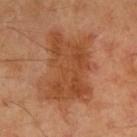Impression: Imaged during a routine full-body skin examination; the lesion was not biopsied and no histopathology is available. Background: A male patient approximately 45 years of age. The total-body-photography lesion software estimated a lesion area of about 35 mm². The software also gave a lesion–skin lightness drop of about 9 and a normalized border contrast of about 7. It also reported a border-irregularity index near 5.5/10, a color-variation rating of about 4/10, and radial color variation of about 1.5. The software also gave a classifier nevus-likeness of about 0/100 and lesion-presence confidence of about 100/100. Captured under cross-polarized illumination. A 15 mm close-up tile from a total-body photography series done for melanoma screening. On the right upper arm. The recorded lesion diameter is about 8 mm.